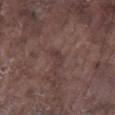On the right lower leg.
The tile uses white-light illumination.
A male patient, aged 73 to 77.
Cropped from a total-body skin-imaging series; the visible field is about 15 mm.